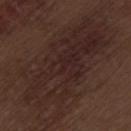The recorded lesion diameter is about 15 mm.
A 15 mm close-up tile from a total-body photography series done for melanoma screening.
The patient is a male roughly 70 years of age.
Located on the leg.
Automated tile analysis of the lesion measured a color-variation rating of about 4.5/10 and peripheral color asymmetry of about 1.5.
Captured under white-light illumination.